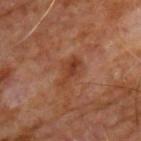Assessment:
No biopsy was performed on this lesion — it was imaged during a full skin examination and was not determined to be concerning.
Context:
A male patient roughly 60 years of age. The lesion's longest dimension is about 3.5 mm. Imaged with cross-polarized lighting. The lesion is on the chest. The total-body-photography lesion software estimated a footprint of about 6 mm² and an outline eccentricity of about 0.8 (0 = round, 1 = elongated). Cropped from a total-body skin-imaging series; the visible field is about 15 mm.